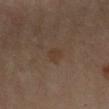Imaged with cross-polarized lighting.
Longest diameter approximately 2.5 mm.
A female subject aged 58–62.
The total-body-photography lesion software estimated a footprint of about 3.5 mm², an outline eccentricity of about 0.75 (0 = round, 1 = elongated), and two-axis asymmetry of about 0.35. It also reported a mean CIELAB color near L≈36 a*≈15 b*≈25, about 5 CIELAB-L* units darker than the surrounding skin, and a normalized border contrast of about 5.
A 15 mm close-up extracted from a 3D total-body photography capture.
The lesion is located on the left forearm.